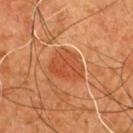{"biopsy_status": "not biopsied; imaged during a skin examination", "automated_metrics": {"area_mm2_approx": 11.0, "eccentricity": 0.65, "shape_asymmetry": 0.2, "vs_skin_darker_L": 8.0, "vs_skin_contrast_norm": 6.0}, "patient": {"sex": "male", "age_approx": 55}, "lighting": "cross-polarized", "lesion_size": {"long_diameter_mm_approx": 4.5}, "image": {"source": "total-body photography crop", "field_of_view_mm": 15}, "site": "chest"}